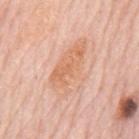Q: Was a biopsy performed?
A: imaged on a skin check; not biopsied
Q: What is the anatomic site?
A: the mid back
Q: Illumination type?
A: white-light
Q: What is the imaging modality?
A: ~15 mm tile from a whole-body skin photo
Q: Automated lesion metrics?
A: a footprint of about 17 mm², an outline eccentricity of about 0.9 (0 = round, 1 = elongated), and a shape-asymmetry score of about 0.25 (0 = symmetric)
Q: Who is the patient?
A: male, in their 80s